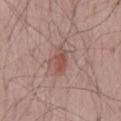The lesion is on the mid back. A 15 mm close-up extracted from a 3D total-body photography capture. A male subject, aged 63 to 67.